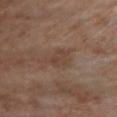biopsy status: imaged on a skin check; not biopsied
body site: the left lower leg
illumination: cross-polarized illumination
image-analysis metrics: a lesion area of about 4.5 mm² and two-axis asymmetry of about 0.4; an average lesion color of about L≈39 a*≈16 b*≈24 (CIELAB) and a lesion-to-skin contrast of about 5 (normalized; higher = more distinct); a nevus-likeness score of about 0/100 and lesion-presence confidence of about 100/100
imaging modality: ~15 mm crop, total-body skin-cancer survey
subject: male, roughly 70 years of age